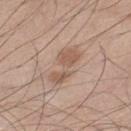  biopsy_status: not biopsied; imaged during a skin examination
  patient:
    sex: male
    age_approx: 45
  site: left lower leg
  image:
    source: total-body photography crop
    field_of_view_mm: 15
  lighting: white-light
  lesion_size:
    long_diameter_mm_approx: 5.0